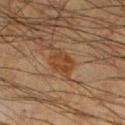Imaged during a routine full-body skin examination; the lesion was not biopsied and no histopathology is available. The subject is a male approximately 35 years of age. A lesion tile, about 15 mm wide, cut from a 3D total-body photograph. The lesion is on the left lower leg.